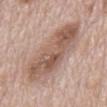  biopsy_status: not biopsied; imaged during a skin examination
  lesion_size:
    long_diameter_mm_approx: 11.0
  site: mid back
  automated_metrics:
    area_mm2_approx: 33.0
    eccentricity: 0.95
    shape_asymmetry: 0.2
    cielab_L: 56
    cielab_a: 18
    cielab_b: 26
    vs_skin_darker_L: 12.0
    vs_skin_contrast_norm: 8.0
    border_irregularity_0_10: 3.5
    color_variation_0_10: 7.0
    peripheral_color_asymmetry: 3.0
  patient:
    sex: male
    age_approx: 70
  image:
    source: total-body photography crop
    field_of_view_mm: 15
  lighting: white-light